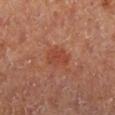Imaged during a routine full-body skin examination; the lesion was not biopsied and no histopathology is available. From the right lower leg. The patient is female. Measured at roughly 3.5 mm in maximum diameter. This is a cross-polarized tile. An algorithmic analysis of the crop reported an outline eccentricity of about 0.8 (0 = round, 1 = elongated) and a symmetry-axis asymmetry near 0.25. The analysis additionally found a mean CIELAB color near L≈46 a*≈29 b*≈34 and a lesion–skin lightness drop of about 7. And it measured a border-irregularity index near 2.5/10, a within-lesion color-variation index near 3/10, and radial color variation of about 1. A 15 mm close-up tile from a total-body photography series done for melanoma screening.